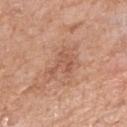Q: Was a biopsy performed?
A: total-body-photography surveillance lesion; no biopsy
Q: What is the anatomic site?
A: the right upper arm
Q: What kind of image is this?
A: 15 mm crop, total-body photography
Q: Who is the patient?
A: female, roughly 75 years of age